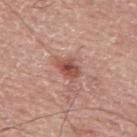The lesion was tiled from a total-body skin photograph and was not biopsied. The tile uses white-light illumination. A region of skin cropped from a whole-body photographic capture, roughly 15 mm wide. The patient is a male aged around 75. Located on the back.